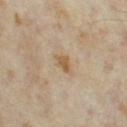location: the leg; image source: 15 mm crop, total-body photography; size: about 3 mm; subject: female, aged around 35; illumination: cross-polarized illumination.